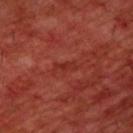Case summary:
– diameter: about 2.5 mm
– lighting: cross-polarized
– location: the upper back
– acquisition: total-body-photography crop, ~15 mm field of view
– patient: male, aged 58 to 62